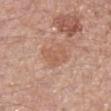<case>
  <lesion_size>
    <long_diameter_mm_approx>3.5</long_diameter_mm_approx>
  </lesion_size>
  <patient>
    <sex>female</sex>
    <age_approx>50</age_approx>
  </patient>
  <lighting>white-light</lighting>
  <image>
    <source>total-body photography crop</source>
    <field_of_view_mm>15</field_of_view_mm>
  </image>
  <site>left forearm</site>
  <automated_metrics>
    <nevus_likeness_0_100>20</nevus_likeness_0_100>
    <lesion_detection_confidence_0_100>100</lesion_detection_confidence_0_100>
  </automated_metrics>
</case>